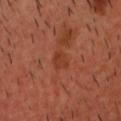Findings:
– follow-up · total-body-photography surveillance lesion; no biopsy
– subject · male, in their 40s
– anatomic site · the head or neck
– lesion size · ≈2.5 mm
– lighting · cross-polarized
– automated metrics · a lesion color around L≈38 a*≈28 b*≈33 in CIELAB and roughly 6 lightness units darker than nearby skin
– imaging modality · ~15 mm tile from a whole-body skin photo